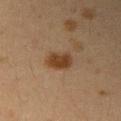The lesion was tiled from a total-body skin photograph and was not biopsied.
A region of skin cropped from a whole-body photographic capture, roughly 15 mm wide.
A female subject, approximately 40 years of age.
About 3.5 mm across.
On the right upper arm.
Captured under cross-polarized illumination.